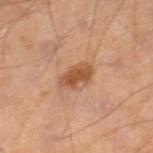Notes:
- workup: catalogued during a skin exam; not biopsied
- image source: 15 mm crop, total-body photography
- patient: male, in their mid- to late 40s
- anatomic site: the right lower leg